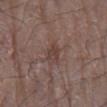Approximately 3 mm at its widest.
A male subject, approximately 80 years of age.
Cropped from a whole-body photographic skin survey; the tile spans about 15 mm.
The lesion is on the right arm.